A roughly 15 mm field-of-view crop from a total-body skin photograph · The total-body-photography lesion software estimated an average lesion color of about L≈29 a*≈23 b*≈23 (CIELAB), roughly 9 lightness units darker than nearby skin, and a normalized border contrast of about 9. And it measured a border-irregularity index near 5/10, internal color variation of about 4 on a 0–10 scale, and peripheral color asymmetry of about 1.5. And it measured a nevus-likeness score of about 25/100 and lesion-presence confidence of about 100/100 · a male patient, aged 53–57 · this is a cross-polarized tile · from the head or neck · about 5 mm across:
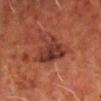On excision, pathology confirmed a nodular basal cell carcinoma, classified as a malignant lesion.